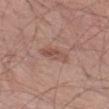Clinical impression:
No biopsy was performed on this lesion — it was imaged during a full skin examination and was not determined to be concerning.
Background:
A close-up tile cropped from a whole-body skin photograph, about 15 mm across. A male patient, aged 68–72. On the left thigh.